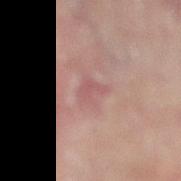| feature | finding |
|---|---|
| biopsy status | imaged on a skin check; not biopsied |
| image-analysis metrics | an area of roughly 3.5 mm², a shape eccentricity near 0.75, and two-axis asymmetry of about 0.5; a lesion color around L≈48 a*≈21 b*≈19 in CIELAB, about 6 CIELAB-L* units darker than the surrounding skin, and a lesion-to-skin contrast of about 4.5 (normalized; higher = more distinct); an automated nevus-likeness rating near 0 out of 100 |
| diameter | about 3 mm |
| imaging modality | 15 mm crop, total-body photography |
| patient | male, in their 60s |
| lighting | cross-polarized |
| body site | the right lower leg |